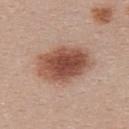{
  "biopsy_status": "not biopsied; imaged during a skin examination",
  "site": "upper back",
  "automated_metrics": {
    "vs_skin_darker_L": 15.0,
    "nevus_likeness_0_100": 100
  },
  "lesion_size": {
    "long_diameter_mm_approx": 7.0
  },
  "patient": {
    "sex": "female",
    "age_approx": 25
  },
  "image": {
    "source": "total-body photography crop",
    "field_of_view_mm": 15
  }
}